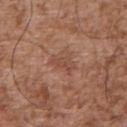<record>
<biopsy_status>not biopsied; imaged during a skin examination</biopsy_status>
<lighting>white-light</lighting>
<patient>
  <sex>male</sex>
  <age_approx>55</age_approx>
</patient>
<image>
  <source>total-body photography crop</source>
  <field_of_view_mm>15</field_of_view_mm>
</image>
<automated_metrics>
  <cielab_L>47</cielab_L>
  <cielab_a>23</cielab_a>
  <cielab_b>28</cielab_b>
  <vs_skin_darker_L>7.0</vs_skin_darker_L>
  <vs_skin_contrast_norm>5.0</vs_skin_contrast_norm>
  <border_irregularity_0_10>3.5</border_irregularity_0_10>
  <color_variation_0_10>2.0</color_variation_0_10>
  <peripheral_color_asymmetry>0.5</peripheral_color_asymmetry>
</automated_metrics>
<site>back</site>
</record>